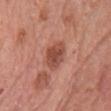notes = imaged on a skin check; not biopsied
acquisition = 15 mm crop, total-body photography
patient = female, aged 58–62
lesion diameter = about 3.5 mm
location = the chest
tile lighting = white-light illumination